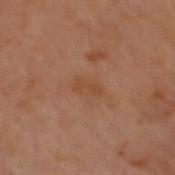Q: Is there a histopathology result?
A: total-body-photography surveillance lesion; no biopsy
Q: Lesion size?
A: ~2.5 mm (longest diameter)
Q: Automated lesion metrics?
A: a footprint of about 2.5 mm² and an outline eccentricity of about 0.9 (0 = round, 1 = elongated); a mean CIELAB color near L≈36 a*≈18 b*≈27, about 4 CIELAB-L* units darker than the surrounding skin, and a lesion-to-skin contrast of about 5 (normalized; higher = more distinct)
Q: Patient demographics?
A: male, approximately 50 years of age
Q: What kind of image is this?
A: total-body-photography crop, ~15 mm field of view
Q: Lesion location?
A: the head or neck